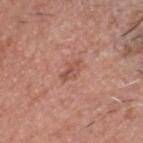A male subject, aged 68 to 72. The lesion is on the head or neck. Captured under white-light illumination. This image is a 15 mm lesion crop taken from a total-body photograph. Automated tile analysis of the lesion measured an automated nevus-likeness rating near 0 out of 100. About 3 mm across.